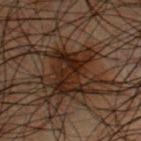Clinical impression: Imaged during a routine full-body skin examination; the lesion was not biopsied and no histopathology is available. Context: The patient is a male in their 50s. Cropped from a whole-body photographic skin survey; the tile spans about 15 mm. Located on the chest. Captured under cross-polarized illumination.